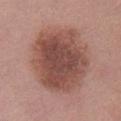Captured during whole-body skin photography for melanoma surveillance; the lesion was not biopsied.
The total-body-photography lesion software estimated a lesion area of about 43 mm², an eccentricity of roughly 0.55, and a symmetry-axis asymmetry near 0.1. The analysis additionally found a border-irregularity index near 2/10 and a within-lesion color-variation index near 4.5/10.
A female patient aged 48–52.
Located on the chest.
Cropped from a whole-body photographic skin survey; the tile spans about 15 mm.
Approximately 8.5 mm at its widest.